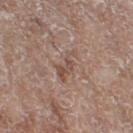No biopsy was performed on this lesion — it was imaged during a full skin examination and was not determined to be concerning. Cropped from a total-body skin-imaging series; the visible field is about 15 mm. Longest diameter approximately 4 mm. From the right thigh. The patient is a female aged approximately 75. Captured under white-light illumination.